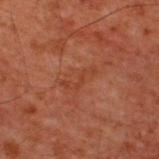Case summary:
- notes — no biopsy performed (imaged during a skin exam)
- image — 15 mm crop, total-body photography
- TBP lesion metrics — a footprint of about 3 mm² and two-axis asymmetry of about 0.65
- subject — male, aged around 60
- tile lighting — cross-polarized
- diameter — ≈3.5 mm
- location — the upper back